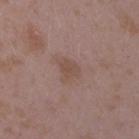No biopsy was performed on this lesion — it was imaged during a full skin examination and was not determined to be concerning. On the arm. A female subject aged 33 to 37. The total-body-photography lesion software estimated a classifier nevus-likeness of about 0/100 and a lesion-detection confidence of about 100/100. Cropped from a whole-body photographic skin survey; the tile spans about 15 mm. Imaged with white-light lighting.